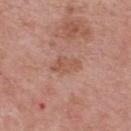Captured during whole-body skin photography for melanoma surveillance; the lesion was not biopsied. Approximately 3.5 mm at its widest. A male patient, in their mid- to late 60s. On the upper back. This image is a 15 mm lesion crop taken from a total-body photograph. Imaged with white-light lighting.